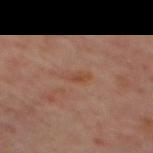Assessment: The lesion was photographed on a routine skin check and not biopsied; there is no pathology result. Image and clinical context: On the mid back. Measured at roughly 3 mm in maximum diameter. A roughly 15 mm field-of-view crop from a total-body skin photograph. The lesion-visualizer software estimated a mean CIELAB color near L≈45 a*≈20 b*≈29, a lesion–skin lightness drop of about 5, and a lesion-to-skin contrast of about 5.5 (normalized; higher = more distinct). And it measured a border-irregularity index near 3/10. This is a cross-polarized tile. A male patient aged 63 to 67.